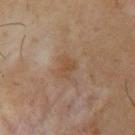workup — catalogued during a skin exam; not biopsied | imaging modality — ~15 mm tile from a whole-body skin photo | body site — the upper back | size — ~2.5 mm (longest diameter) | automated metrics — a lesion area of about 4 mm², an outline eccentricity of about 0.75 (0 = round, 1 = elongated), and a symmetry-axis asymmetry near 0.25; a mean CIELAB color near L≈45 a*≈16 b*≈29 and a normalized border contrast of about 6; border irregularity of about 3 on a 0–10 scale and internal color variation of about 2.5 on a 0–10 scale; a classifier nevus-likeness of about 0/100 and a lesion-detection confidence of about 100/100 | patient — male, in their mid- to late 60s.